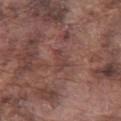workup=catalogued during a skin exam; not biopsied | illumination=white-light illumination | location=the left thigh | image=~15 mm tile from a whole-body skin photo | diameter=≈3 mm | patient=male, approximately 75 years of age.